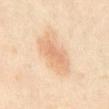Findings:
- follow-up: total-body-photography surveillance lesion; no biopsy
- anatomic site: the abdomen
- subject: male, roughly 50 years of age
- image source: ~15 mm tile from a whole-body skin photo